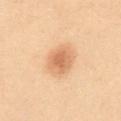notes: no biopsy performed (imaged during a skin exam) | location: the abdomen | image source: ~15 mm tile from a whole-body skin photo | lighting: cross-polarized illumination | automated lesion analysis: an average lesion color of about L≈54 a*≈18 b*≈32 (CIELAB), a lesion–skin lightness drop of about 9, and a lesion-to-skin contrast of about 6.5 (normalized; higher = more distinct) | lesion diameter: about 3.5 mm | subject: female, about 40 years old.